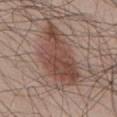Impression: Recorded during total-body skin imaging; not selected for excision or biopsy. Acquisition and patient details: A 15 mm close-up tile from a total-body photography series done for melanoma screening. Longest diameter approximately 9 mm. The lesion is located on the abdomen. This is a white-light tile. An algorithmic analysis of the crop reported border irregularity of about 4.5 on a 0–10 scale, a within-lesion color-variation index near 5.5/10, and peripheral color asymmetry of about 2. And it measured an automated nevus-likeness rating near 95 out of 100 and a lesion-detection confidence of about 100/100. A male patient, roughly 50 years of age.